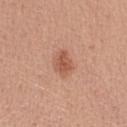The lesion was tiled from a total-body skin photograph and was not biopsied.